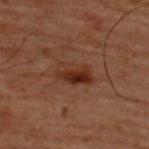| field | value |
|---|---|
| notes | total-body-photography surveillance lesion; no biopsy |
| tile lighting | cross-polarized illumination |
| patient | male, aged around 60 |
| diameter | ~4 mm (longest diameter) |
| body site | the back |
| image source | total-body-photography crop, ~15 mm field of view |